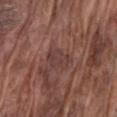{
  "biopsy_status": "not biopsied; imaged during a skin examination",
  "image": {
    "source": "total-body photography crop",
    "field_of_view_mm": 15
  },
  "patient": {
    "sex": "male",
    "age_approx": 75
  },
  "automated_metrics": {
    "area_mm2_approx": 6.0,
    "eccentricity": 0.8,
    "shape_asymmetry": 0.3,
    "border_irregularity_0_10": 3.5,
    "color_variation_0_10": 2.0,
    "peripheral_color_asymmetry": 0.5,
    "nevus_likeness_0_100": 0,
    "lesion_detection_confidence_0_100": 90
  },
  "lighting": "white-light",
  "lesion_size": {
    "long_diameter_mm_approx": 3.5
  },
  "site": "left upper arm"
}